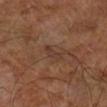automated lesion analysis — a lesion–skin lightness drop of about 6 and a lesion-to-skin contrast of about 5.5 (normalized; higher = more distinct); a border-irregularity index near 4/10, internal color variation of about 0.5 on a 0–10 scale, and a peripheral color-asymmetry measure near 0; a nevus-likeness score of about 0/100
patient — aged approximately 65
anatomic site — the right lower leg
acquisition — 15 mm crop, total-body photography
size — ~3 mm (longest diameter)